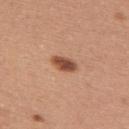workup: catalogued during a skin exam; not biopsied
automated metrics: a lesion color around L≈50 a*≈24 b*≈32 in CIELAB and roughly 15 lightness units darker than nearby skin; a border-irregularity rating of about 2/10, internal color variation of about 3.5 on a 0–10 scale, and radial color variation of about 1
acquisition: ~15 mm tile from a whole-body skin photo
anatomic site: the upper back
patient: female, in their mid-30s
lesion diameter: about 3 mm